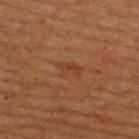Clinical impression: No biopsy was performed on this lesion — it was imaged during a full skin examination and was not determined to be concerning. Context: A 15 mm crop from a total-body photograph taken for skin-cancer surveillance. A male subject, in their mid- to late 50s. The lesion is located on the upper back. Approximately 3 mm at its widest.